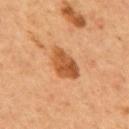| feature | finding |
|---|---|
| biopsy status | catalogued during a skin exam; not biopsied |
| lesion diameter | ~4.5 mm (longest diameter) |
| image source | 15 mm crop, total-body photography |
| subject | male, roughly 55 years of age |
| automated metrics | a lesion color around L≈50 a*≈24 b*≈39 in CIELAB, about 12 CIELAB-L* units darker than the surrounding skin, and a normalized lesion–skin contrast near 9; a border-irregularity rating of about 2.5/10, a within-lesion color-variation index near 5/10, and a peripheral color-asymmetry measure near 2 |
| anatomic site | the back |